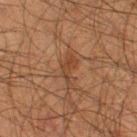Imaged during a routine full-body skin examination; the lesion was not biopsied and no histopathology is available. This is a cross-polarized tile. A male subject, approximately 35 years of age. A 15 mm close-up extracted from a 3D total-body photography capture. The total-body-photography lesion software estimated a border-irregularity rating of about 6.5/10 and internal color variation of about 1.5 on a 0–10 scale. The software also gave a classifier nevus-likeness of about 0/100 and a detector confidence of about 90 out of 100 that the crop contains a lesion. The lesion is on the right thigh.